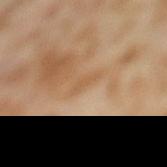biopsy_status: not biopsied; imaged during a skin examination
site: left thigh
patient:
  sex: female
  age_approx: 55
image:
  source: total-body photography crop
  field_of_view_mm: 15
lesion_size:
  long_diameter_mm_approx: 2.5
automated_metrics:
  area_mm2_approx: 2.0
  eccentricity: 0.9
  shape_asymmetry: 0.45
  color_variation_0_10: 0.0
  peripheral_color_asymmetry: 0.0
  nevus_likeness_0_100: 0